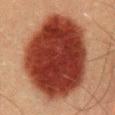Captured during whole-body skin photography for melanoma surveillance; the lesion was not biopsied.
The tile uses cross-polarized illumination.
A 15 mm close-up tile from a total-body photography series done for melanoma screening.
The lesion is located on the abdomen.
A male patient, aged 38–42.